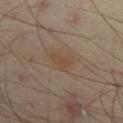Part of a total-body skin-imaging series; this lesion was reviewed on a skin check and was not flagged for biopsy. Longest diameter approximately 2.5 mm. Captured under cross-polarized illumination. The total-body-photography lesion software estimated an area of roughly 4 mm² and two-axis asymmetry of about 0.45. The software also gave an automated nevus-likeness rating near 5 out of 100 and a lesion-detection confidence of about 100/100. From the right thigh. The subject is a male in their mid-50s. This image is a 15 mm lesion crop taken from a total-body photograph.